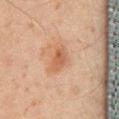<case>
  <biopsy_status>not biopsied; imaged during a skin examination</biopsy_status>
  <image>
    <source>total-body photography crop</source>
    <field_of_view_mm>15</field_of_view_mm>
  </image>
  <lesion_size>
    <long_diameter_mm_approx>3.5</long_diameter_mm_approx>
  </lesion_size>
  <automated_metrics>
    <border_irregularity_0_10>2.5</border_irregularity_0_10>
    <color_variation_0_10>2.5</color_variation_0_10>
    <peripheral_color_asymmetry>1.0</peripheral_color_asymmetry>
    <lesion_detection_confidence_0_100>100</lesion_detection_confidence_0_100>
  </automated_metrics>
  <site>back</site>
  <patient>
    <sex>male</sex>
    <age_approx>45</age_approx>
  </patient>
  <lighting>cross-polarized</lighting>
</case>